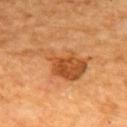{"biopsy_status": "not biopsied; imaged during a skin examination", "site": "mid back", "patient": {"sex": "male", "age_approx": 60}, "lesion_size": {"long_diameter_mm_approx": 8.5}, "automated_metrics": {"area_mm2_approx": 18.0, "eccentricity": 0.9, "cielab_L": 48, "cielab_a": 25, "cielab_b": 40, "vs_skin_darker_L": 11.0, "vs_skin_contrast_norm": 8.0, "nevus_likeness_0_100": 90, "lesion_detection_confidence_0_100": 100}, "lighting": "cross-polarized", "image": {"source": "total-body photography crop", "field_of_view_mm": 15}}